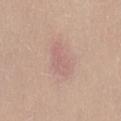Q: Was this lesion biopsied?
A: total-body-photography surveillance lesion; no biopsy
Q: What is the lesion's diameter?
A: ≈4 mm
Q: How was this image acquired?
A: total-body-photography crop, ~15 mm field of view
Q: What lighting was used for the tile?
A: white-light illumination
Q: Patient demographics?
A: female, aged 28–32
Q: What is the anatomic site?
A: the back